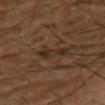Q: Is there a histopathology result?
A: no biopsy performed (imaged during a skin exam)
Q: Lesion size?
A: ~3.5 mm (longest diameter)
Q: What lighting was used for the tile?
A: cross-polarized illumination
Q: What kind of image is this?
A: total-body-photography crop, ~15 mm field of view
Q: Patient demographics?
A: male, in their 60s
Q: Where on the body is the lesion?
A: the right arm